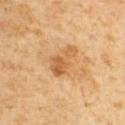Q: Was this lesion biopsied?
A: catalogued during a skin exam; not biopsied
Q: What are the patient's age and sex?
A: male, roughly 65 years of age
Q: Illumination type?
A: cross-polarized
Q: What did automated image analysis measure?
A: a color-variation rating of about 2.5/10 and a peripheral color-asymmetry measure near 0.5
Q: What kind of image is this?
A: 15 mm crop, total-body photography